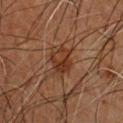A 15 mm close-up extracted from a 3D total-body photography capture. From the chest. A male patient aged 63–67.